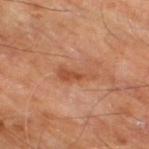The lesion was tiled from a total-body skin photograph and was not biopsied.
The lesion is on the leg.
The patient is aged 63 to 67.
The lesion-visualizer software estimated an outline eccentricity of about 0.95 (0 = round, 1 = elongated) and a shape-asymmetry score of about 0.4 (0 = symmetric).
The lesion's longest dimension is about 4.5 mm.
A roughly 15 mm field-of-view crop from a total-body skin photograph.